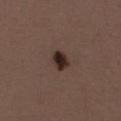Impression:
No biopsy was performed on this lesion — it was imaged during a full skin examination and was not determined to be concerning.
Image and clinical context:
A female patient aged 48–52. About 2.5 mm across. From the abdomen. Automated tile analysis of the lesion measured a lesion area of about 4.5 mm², an outline eccentricity of about 0.65 (0 = round, 1 = elongated), and a shape-asymmetry score of about 0.25 (0 = symmetric). It also reported a lesion color around L≈27 a*≈15 b*≈19 in CIELAB and a lesion-to-skin contrast of about 13 (normalized; higher = more distinct). It also reported a border-irregularity rating of about 2/10 and a peripheral color-asymmetry measure near 1.5. Captured under white-light illumination. A close-up tile cropped from a whole-body skin photograph, about 15 mm across.